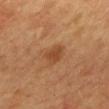Q: Is there a histopathology result?
A: total-body-photography surveillance lesion; no biopsy
Q: Automated lesion metrics?
A: a lesion area of about 5 mm², an eccentricity of roughly 0.55, and two-axis asymmetry of about 0.25; a mean CIELAB color near L≈40 a*≈21 b*≈33 and a lesion–skin lightness drop of about 7; a classifier nevus-likeness of about 15/100
Q: Lesion location?
A: the mid back
Q: How large is the lesion?
A: about 2.5 mm
Q: How was the tile lit?
A: cross-polarized illumination
Q: Patient demographics?
A: female, aged approximately 60
Q: What kind of image is this?
A: total-body-photography crop, ~15 mm field of view